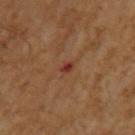– workup · imaged on a skin check; not biopsied
– imaging modality · total-body-photography crop, ~15 mm field of view
– subject · male, aged 58–62
– location · the left upper arm